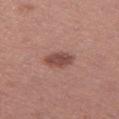| field | value |
|---|---|
| workup | catalogued during a skin exam; not biopsied |
| size | ~3.5 mm (longest diameter) |
| patient | female, aged approximately 40 |
| image | ~15 mm crop, total-body skin-cancer survey |
| location | the left thigh |
| lighting | white-light |